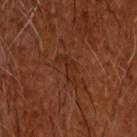This lesion was catalogued during total-body skin photography and was not selected for biopsy. Approximately 4 mm at its widest. A male subject, aged around 65. A 15 mm close-up extracted from a 3D total-body photography capture. The lesion-visualizer software estimated an area of roughly 4.5 mm², an eccentricity of roughly 0.9, and a symmetry-axis asymmetry near 0.6. The software also gave a border-irregularity index near 8/10 and a within-lesion color-variation index near 0/10.